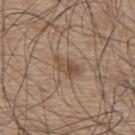Approximately 4 mm at its widest. Automated tile analysis of the lesion measured a footprint of about 6 mm², an outline eccentricity of about 0.85 (0 = round, 1 = elongated), and a shape-asymmetry score of about 0.35 (0 = symmetric). The analysis additionally found a classifier nevus-likeness of about 10/100 and a lesion-detection confidence of about 100/100. On the mid back. A male subject aged approximately 75. This image is a 15 mm lesion crop taken from a total-body photograph. The tile uses white-light illumination.